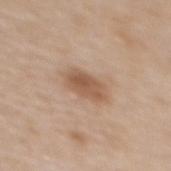Q: Was a biopsy performed?
A: no biopsy performed (imaged during a skin exam)
Q: How was the tile lit?
A: white-light
Q: What is the anatomic site?
A: the upper back
Q: What are the patient's age and sex?
A: female, aged 63–67
Q: What kind of image is this?
A: total-body-photography crop, ~15 mm field of view